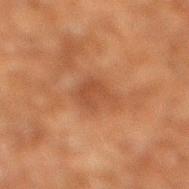Imaged during a routine full-body skin examination; the lesion was not biopsied and no histopathology is available. The lesion's longest dimension is about 3.5 mm. Imaged with cross-polarized lighting. The subject is a male in their 60s. This image is a 15 mm lesion crop taken from a total-body photograph. The lesion is on the right lower leg.